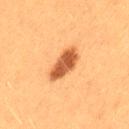  biopsy_status: not biopsied; imaged during a skin examination
  patient:
    sex: female
    age_approx: 40
  site: left thigh
  lighting: cross-polarized
  image:
    source: total-body photography crop
    field_of_view_mm: 15
  automated_metrics:
    border_irregularity_0_10: 2.0
    color_variation_0_10: 3.5
    peripheral_color_asymmetry: 1.0
    nevus_likeness_0_100: 100
    lesion_detection_confidence_0_100: 100